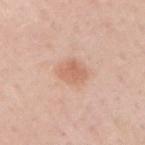Notes:
* biopsy status: catalogued during a skin exam; not biopsied
* anatomic site: the arm
* acquisition: total-body-photography crop, ~15 mm field of view
* subject: male, aged 58–62
* illumination: white-light